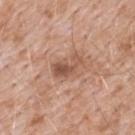location — the upper back; subject — male, approximately 50 years of age; acquisition — total-body-photography crop, ~15 mm field of view.